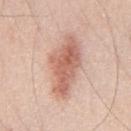| field | value |
|---|---|
| lighting | white-light illumination |
| acquisition | total-body-photography crop, ~15 mm field of view |
| patient | male, roughly 45 years of age |
| image-analysis metrics | an eccentricity of roughly 0.9 and a symmetry-axis asymmetry near 0.25; a classifier nevus-likeness of about 65/100 and lesion-presence confidence of about 100/100 |
| lesion diameter | about 8 mm |
| location | the chest |